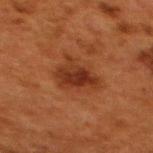This lesion was catalogued during total-body skin photography and was not selected for biopsy. About 5 mm across. Located on the upper back. The tile uses cross-polarized illumination. A lesion tile, about 15 mm wide, cut from a 3D total-body photograph. An algorithmic analysis of the crop reported a mean CIELAB color near L≈29 a*≈23 b*≈29 and a normalized border contrast of about 7.5. And it measured a border-irregularity rating of about 3.5/10, internal color variation of about 4.5 on a 0–10 scale, and a peripheral color-asymmetry measure near 1.5. The analysis additionally found a nevus-likeness score of about 50/100 and a lesion-detection confidence of about 100/100. A female patient, approximately 50 years of age.